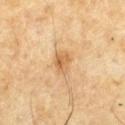| feature | finding |
|---|---|
| workup | catalogued during a skin exam; not biopsied |
| subject | male, in their mid- to late 60s |
| acquisition | 15 mm crop, total-body photography |
| automated metrics | a footprint of about 4 mm² and an eccentricity of roughly 0.7; a lesion color around L≈56 a*≈18 b*≈38 in CIELAB and a lesion-to-skin contrast of about 6.5 (normalized; higher = more distinct); a border-irregularity index near 2.5/10, a color-variation rating of about 3.5/10, and peripheral color asymmetry of about 1.5 |
| lighting | cross-polarized |
| site | the chest |
| diameter | about 2.5 mm |